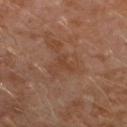biopsy status = imaged on a skin check; not biopsied
imaging modality = ~15 mm crop, total-body skin-cancer survey
patient = male, aged around 30
tile lighting = cross-polarized illumination
body site = the leg